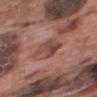  biopsy_status: not biopsied; imaged during a skin examination
  lighting: white-light
  patient:
    sex: male
    age_approx: 70
  site: upper back
  image:
    source: total-body photography crop
    field_of_view_mm: 15
  lesion_size:
    long_diameter_mm_approx: 4.0
  automated_metrics:
    cielab_L: 46
    cielab_a: 21
    cielab_b: 26
    vs_skin_darker_L: 9.0
    vs_skin_contrast_norm: 7.0
    border_irregularity_0_10: 2.5
    color_variation_0_10: 5.0
    nevus_likeness_0_100: 0
    lesion_detection_confidence_0_100: 100